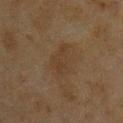A male patient approximately 45 years of age.
Measured at roughly 3.5 mm in maximum diameter.
Imaged with cross-polarized lighting.
A lesion tile, about 15 mm wide, cut from a 3D total-body photograph.
The lesion is located on the back.